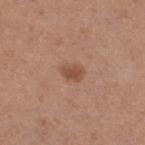Recorded during total-body skin imaging; not selected for excision or biopsy.
On the right thigh.
Measured at roughly 2.5 mm in maximum diameter.
A 15 mm close-up extracted from a 3D total-body photography capture.
The patient is a female aged around 40.
Captured under white-light illumination.